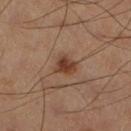notes — no biopsy performed (imaged during a skin exam); anatomic site — the right lower leg; imaging modality — ~15 mm tile from a whole-body skin photo; subject — male, about 60 years old.